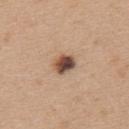Q: Was a biopsy performed?
A: total-body-photography surveillance lesion; no biopsy
Q: What are the patient's age and sex?
A: female, about 45 years old
Q: What is the anatomic site?
A: the back
Q: How was this image acquired?
A: ~15 mm tile from a whole-body skin photo
Q: What did automated image analysis measure?
A: a lesion color around L≈47 a*≈19 b*≈27 in CIELAB and roughly 19 lightness units darker than nearby skin
Q: How was the tile lit?
A: white-light illumination
Q: Lesion size?
A: about 2.5 mm